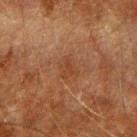Captured during whole-body skin photography for melanoma surveillance; the lesion was not biopsied.
Imaged with cross-polarized lighting.
The lesion's longest dimension is about 2.5 mm.
Located on the arm.
Cropped from a whole-body photographic skin survey; the tile spans about 15 mm.
A male patient about 60 years old.
The lesion-visualizer software estimated an outline eccentricity of about 0.65 (0 = round, 1 = elongated) and two-axis asymmetry of about 0.4. The software also gave a mean CIELAB color near L≈32 a*≈18 b*≈28, about 5 CIELAB-L* units darker than the surrounding skin, and a normalized lesion–skin contrast near 5.